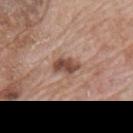{"biopsy_status": "not biopsied; imaged during a skin examination", "site": "mid back", "image": {"source": "total-body photography crop", "field_of_view_mm": 15}, "lesion_size": {"long_diameter_mm_approx": 3.0}, "lighting": "white-light", "automated_metrics": {"eccentricity": 0.75, "shape_asymmetry": 0.2, "border_irregularity_0_10": 2.5, "color_variation_0_10": 4.0, "peripheral_color_asymmetry": 1.5}, "patient": {"sex": "male", "age_approx": 70}}